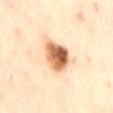This image is a 15 mm lesion crop taken from a total-body photograph. Approximately 4.5 mm at its widest. Imaged with cross-polarized lighting. The lesion is located on the back. A male subject aged 53–57. An algorithmic analysis of the crop reported an average lesion color of about L≈64 a*≈22 b*≈37 (CIELAB), roughly 21 lightness units darker than nearby skin, and a normalized lesion–skin contrast near 12. The analysis additionally found a border-irregularity index near 2.5/10 and peripheral color asymmetry of about 3.5. It also reported a classifier nevus-likeness of about 100/100 and lesion-presence confidence of about 100/100.